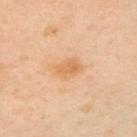This lesion was catalogued during total-body skin photography and was not selected for biopsy. A region of skin cropped from a whole-body photographic capture, roughly 15 mm wide. The total-body-photography lesion software estimated an average lesion color of about L≈68 a*≈22 b*≈42 (CIELAB) and a normalized border contrast of about 6. It also reported border irregularity of about 2 on a 0–10 scale. The analysis additionally found a detector confidence of about 100 out of 100 that the crop contains a lesion. The lesion's longest dimension is about 3 mm. The patient is a female approximately 40 years of age. Captured under cross-polarized illumination. Located on the left upper arm.